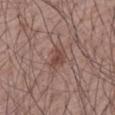{"biopsy_status": "not biopsied; imaged during a skin examination", "lighting": "white-light", "site": "right forearm", "patient": {"sex": "male", "age_approx": 50}, "image": {"source": "total-body photography crop", "field_of_view_mm": 15}, "lesion_size": {"long_diameter_mm_approx": 2.5}}